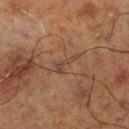Q: What is the lesion's diameter?
A: ~3 mm (longest diameter)
Q: Patient demographics?
A: male, aged approximately 70
Q: What is the anatomic site?
A: the right lower leg
Q: What kind of image is this?
A: total-body-photography crop, ~15 mm field of view